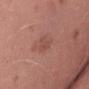Q: Is there a histopathology result?
A: total-body-photography surveillance lesion; no biopsy
Q: What lighting was used for the tile?
A: white-light
Q: How large is the lesion?
A: ~3 mm (longest diameter)
Q: What did automated image analysis measure?
A: a border-irregularity index near 4/10, internal color variation of about 1.5 on a 0–10 scale, and peripheral color asymmetry of about 0.5
Q: Lesion location?
A: the lower back
Q: What kind of image is this?
A: total-body-photography crop, ~15 mm field of view
Q: Who is the patient?
A: male, in their 40s